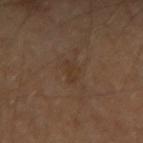| key | value |
|---|---|
| notes | imaged on a skin check; not biopsied |
| acquisition | ~15 mm tile from a whole-body skin photo |
| site | the right upper arm |
| diameter | about 2.5 mm |
| patient | aged 53 to 57 |
| lighting | cross-polarized illumination |